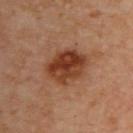<lesion>
<biopsy_status>not biopsied; imaged during a skin examination</biopsy_status>
<lesion_size>
  <long_diameter_mm_approx>4.5</long_diameter_mm_approx>
</lesion_size>
<patient>
  <sex>male</sex>
  <age_approx>65</age_approx>
</patient>
<site>upper back</site>
<image>
  <source>total-body photography crop</source>
  <field_of_view_mm>15</field_of_view_mm>
</image>
<lighting>cross-polarized</lighting>
</lesion>